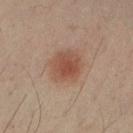Assessment:
Recorded during total-body skin imaging; not selected for excision or biopsy.
Image and clinical context:
Captured under cross-polarized illumination. A male subject, aged 48 to 52. From the right upper arm. This image is a 15 mm lesion crop taken from a total-body photograph. Longest diameter approximately 4 mm.